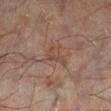follow-up=no biopsy performed (imaged during a skin exam); anatomic site=the left lower leg; image=total-body-photography crop, ~15 mm field of view; automated metrics=a lesion color around L≈43 a*≈16 b*≈25 in CIELAB, a lesion–skin lightness drop of about 5, and a normalized lesion–skin contrast near 5; subject=male, aged 53–57; size=about 3 mm.